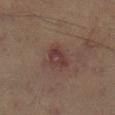workup = no biopsy performed (imaged during a skin exam)
lesion diameter = ~3 mm (longest diameter)
site = the right lower leg
subject = male, in their 60s
lighting = cross-polarized
image source = ~15 mm crop, total-body skin-cancer survey